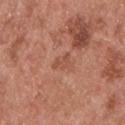{"biopsy_status": "not biopsied; imaged during a skin examination", "lighting": "white-light", "patient": {"sex": "male", "age_approx": 55}, "lesion_size": {"long_diameter_mm_approx": 2.5}, "site": "upper back", "image": {"source": "total-body photography crop", "field_of_view_mm": 15}}